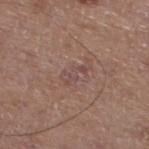Findings:
• follow-up: catalogued during a skin exam; not biopsied
• tile lighting: white-light
• diameter: about 3.5 mm
• automated metrics: a lesion color around L≈47 a*≈19 b*≈21 in CIELAB and a normalized lesion–skin contrast near 5; an automated nevus-likeness rating near 0 out of 100 and a lesion-detection confidence of about 100/100
• location: the left lower leg
• subject: male, aged 63 to 67
• image source: ~15 mm crop, total-body skin-cancer survey